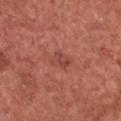Recorded during total-body skin imaging; not selected for excision or biopsy. The subject is a female about 50 years old. Approximately 3 mm at its widest. On the chest. A 15 mm close-up tile from a total-body photography series done for melanoma screening. This is a white-light tile.